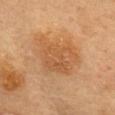  biopsy_status: not biopsied; imaged during a skin examination
  site: mid back
  lesion_size:
    long_diameter_mm_approx: 7.0
  patient:
    sex: female
    age_approx: 60
  image:
    source: total-body photography crop
    field_of_view_mm: 15